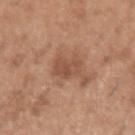Findings:
– follow-up: no biopsy performed (imaged during a skin exam)
– patient: male, aged 38 to 42
– image source: total-body-photography crop, ~15 mm field of view
– site: the left upper arm